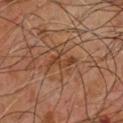No biopsy was performed on this lesion — it was imaged during a full skin examination and was not determined to be concerning. Captured under cross-polarized illumination. Located on the upper back. An algorithmic analysis of the crop reported a classifier nevus-likeness of about 0/100 and a lesion-detection confidence of about 95/100. This image is a 15 mm lesion crop taken from a total-body photograph. A male subject, aged approximately 60.